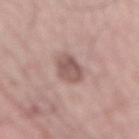<case>
  <biopsy_status>not biopsied; imaged during a skin examination</biopsy_status>
  <lesion_size>
    <long_diameter_mm_approx>4.0</long_diameter_mm_approx>
  </lesion_size>
  <image>
    <source>total-body photography crop</source>
    <field_of_view_mm>15</field_of_view_mm>
  </image>
  <site>mid back</site>
  <automated_metrics>
    <area_mm2_approx>7.0</area_mm2_approx>
    <eccentricity>0.85</eccentricity>
    <shape_asymmetry>0.25</shape_asymmetry>
    <border_irregularity_0_10>2.5</border_irregularity_0_10>
    <color_variation_0_10>3.0</color_variation_0_10>
    <peripheral_color_asymmetry>1.0</peripheral_color_asymmetry>
  </automated_metrics>
  <patient>
    <sex>male</sex>
    <age_approx>65</age_approx>
  </patient>
</case>